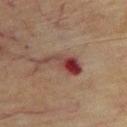Q: Is there a histopathology result?
A: no biopsy performed (imaged during a skin exam)
Q: How was this image acquired?
A: total-body-photography crop, ~15 mm field of view
Q: What are the patient's age and sex?
A: male, aged approximately 75
Q: Lesion location?
A: the upper back
Q: Illumination type?
A: cross-polarized